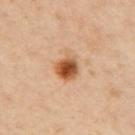The lesion was tiled from a total-body skin photograph and was not biopsied. A roughly 15 mm field-of-view crop from a total-body skin photograph. A female patient, about 40 years old. The lesion is on the left arm.